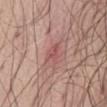Assessment: No biopsy was performed on this lesion — it was imaged during a full skin examination and was not determined to be concerning. Clinical summary: A lesion tile, about 15 mm wide, cut from a 3D total-body photograph. A male subject, aged 68–72. From the front of the torso.